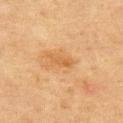{"biopsy_status": "not biopsied; imaged during a skin examination", "patient": {"sex": "male", "age_approx": 65}, "lighting": "cross-polarized", "image": {"source": "total-body photography crop", "field_of_view_mm": 15}, "lesion_size": {"long_diameter_mm_approx": 3.0}, "site": "chest"}